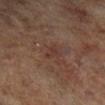biopsy_status: not biopsied; imaged during a skin examination
lesion_size:
  long_diameter_mm_approx: 2.5
image:
  source: total-body photography crop
  field_of_view_mm: 15
site: right lower leg
patient:
  sex: male
  age_approx: 70
lighting: cross-polarized